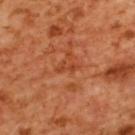No biopsy was performed on this lesion — it was imaged during a full skin examination and was not determined to be concerning.